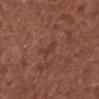Captured during whole-body skin photography for melanoma surveillance; the lesion was not biopsied.
The patient is a male aged 73–77.
Imaged with white-light lighting.
The recorded lesion diameter is about 3 mm.
On the left lower leg.
A roughly 15 mm field-of-view crop from a total-body skin photograph.